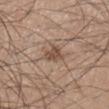The lesion is on the right lower leg. The lesion-visualizer software estimated an average lesion color of about L≈50 a*≈17 b*≈27 (CIELAB), a lesion–skin lightness drop of about 10, and a normalized lesion–skin contrast near 7.5. Measured at roughly 3 mm in maximum diameter. A 15 mm crop from a total-body photograph taken for skin-cancer surveillance. A male subject, about 45 years old. Captured under white-light illumination.